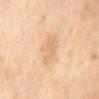Captured during whole-body skin photography for melanoma surveillance; the lesion was not biopsied. Approximately 4 mm at its widest. A close-up tile cropped from a whole-body skin photograph, about 15 mm across. The lesion is on the abdomen. This is a cross-polarized tile. The patient is a female approximately 50 years of age.